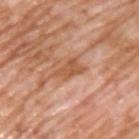Impression:
No biopsy was performed on this lesion — it was imaged during a full skin examination and was not determined to be concerning.
Acquisition and patient details:
The lesion is located on the upper back. This image is a 15 mm lesion crop taken from a total-body photograph. Captured under white-light illumination. A male subject, aged 58–62. Approximately 4.5 mm at its widest.